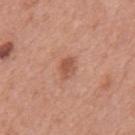Captured during whole-body skin photography for melanoma surveillance; the lesion was not biopsied. Automated image analysis of the tile measured a footprint of about 4 mm², an outline eccentricity of about 0.75 (0 = round, 1 = elongated), and two-axis asymmetry of about 0.25. This is a white-light tile. Approximately 2.5 mm at its widest. The lesion is on the mid back. A close-up tile cropped from a whole-body skin photograph, about 15 mm across. A male subject aged around 55.